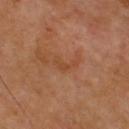The lesion was tiled from a total-body skin photograph and was not biopsied. Captured under cross-polarized illumination. The lesion is on the upper back. A male subject, aged around 70. Cropped from a total-body skin-imaging series; the visible field is about 15 mm. Approximately 3.5 mm at its widest.